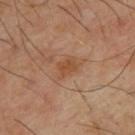<tbp_lesion>
  <biopsy_status>not biopsied; imaged during a skin examination</biopsy_status>
  <patient>
    <sex>male</sex>
    <age_approx>65</age_approx>
  </patient>
  <site>back</site>
  <image>
    <source>total-body photography crop</source>
    <field_of_view_mm>15</field_of_view_mm>
  </image>
</tbp_lesion>